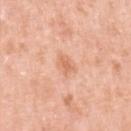notes — imaged on a skin check; not biopsied
TBP lesion metrics — a lesion area of about 4.5 mm² and two-axis asymmetry of about 0.25; border irregularity of about 2.5 on a 0–10 scale and a within-lesion color-variation index near 2/10; an automated nevus-likeness rating near 5 out of 100 and a lesion-detection confidence of about 100/100
subject — female, aged approximately 40
imaging modality — total-body-photography crop, ~15 mm field of view
diameter — ≈2.5 mm
location — the left upper arm
illumination — white-light illumination